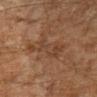Case summary:
– notes: total-body-photography surveillance lesion; no biopsy
– size: ≈4.5 mm
– image: ~15 mm crop, total-body skin-cancer survey
– body site: the right upper arm
– image-analysis metrics: a lesion area of about 10 mm², an eccentricity of roughly 0.75, and two-axis asymmetry of about 0.35; an average lesion color of about L≈32 a*≈16 b*≈25 (CIELAB) and a normalized lesion–skin contrast near 5; a border-irregularity index near 6.5/10, a within-lesion color-variation index near 3/10, and radial color variation of about 0.5
– patient: male, approximately 45 years of age
– illumination: cross-polarized